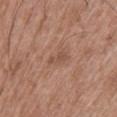Notes:
* image · ~15 mm tile from a whole-body skin photo
* TBP lesion metrics · a nevus-likeness score of about 0/100 and a lesion-detection confidence of about 100/100
* location · the upper back
* illumination · white-light illumination
* patient · male, about 50 years old